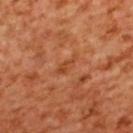<case>
<biopsy_status>not biopsied; imaged during a skin examination</biopsy_status>
<site>upper back</site>
<patient>
  <sex>female</sex>
  <age_approx>55</age_approx>
</patient>
<image>
  <source>total-body photography crop</source>
  <field_of_view_mm>15</field_of_view_mm>
</image>
</case>